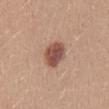Recorded during total-body skin imaging; not selected for excision or biopsy. This is a white-light tile. A female subject aged 23–27. Automated image analysis of the tile measured a footprint of about 9 mm² and a shape eccentricity near 0.65. A 15 mm close-up tile from a total-body photography series done for melanoma screening. The lesion is located on the mid back.